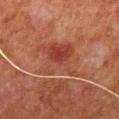The lesion is located on the mid back. About 8 mm across. A close-up tile cropped from a whole-body skin photograph, about 15 mm across. Captured under cross-polarized illumination. A male subject, about 80 years old.